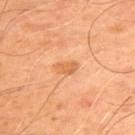{
  "biopsy_status": "not biopsied; imaged during a skin examination",
  "image": {
    "source": "total-body photography crop",
    "field_of_view_mm": 15
  },
  "site": "upper back",
  "patient": {
    "sex": "male",
    "age_approx": 50
  }
}